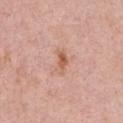Impression: The lesion was tiled from a total-body skin photograph and was not biopsied. Acquisition and patient details: A female subject aged around 30. The lesion is on the chest. Longest diameter approximately 3 mm. This image is a 15 mm lesion crop taken from a total-body photograph. This is a white-light tile.